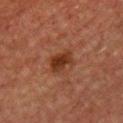- notes: catalogued during a skin exam; not biopsied
- lesion size: ≈3 mm
- anatomic site: the chest
- subject: male, aged approximately 50
- image: ~15 mm crop, total-body skin-cancer survey